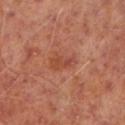Q: Was a biopsy performed?
A: imaged on a skin check; not biopsied
Q: Where on the body is the lesion?
A: the leg
Q: How was this image acquired?
A: ~15 mm crop, total-body skin-cancer survey
Q: Who is the patient?
A: male, aged around 65